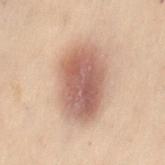biopsy_status: not biopsied; imaged during a skin examination
patient:
  sex: female
  age_approx: 50
site: lower back
image:
  source: total-body photography crop
  field_of_view_mm: 15
lighting: cross-polarized
automated_metrics:
  border_irregularity_0_10: 1.0
  color_variation_0_10: 5.0
  nevus_likeness_0_100: 100